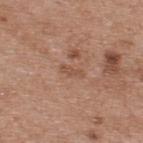Imaged during a routine full-body skin examination; the lesion was not biopsied and no histopathology is available. The tile uses white-light illumination. Cropped from a whole-body photographic skin survey; the tile spans about 15 mm. The lesion-visualizer software estimated an automated nevus-likeness rating near 0 out of 100. On the upper back. A male subject in their mid- to late 50s.